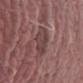Assessment:
The lesion was photographed on a routine skin check and not biopsied; there is no pathology result.
Background:
A male subject aged 43–47. Longest diameter approximately 3.5 mm. On the right thigh. Captured under white-light illumination. This image is a 15 mm lesion crop taken from a total-body photograph. An algorithmic analysis of the crop reported a lesion color around L≈41 a*≈20 b*≈18 in CIELAB. The software also gave an automated nevus-likeness rating near 0 out of 100 and lesion-presence confidence of about 100/100.